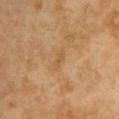| field | value |
|---|---|
| workup | catalogued during a skin exam; not biopsied |